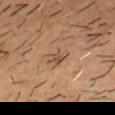The lesion was tiled from a total-body skin photograph and was not biopsied.
A male patient, roughly 50 years of age.
Longest diameter approximately 3 mm.
Cropped from a whole-body photographic skin survey; the tile spans about 15 mm.
The lesion is on the head or neck.
Automated tile analysis of the lesion measured an area of roughly 4.5 mm², an eccentricity of roughly 0.85, and two-axis asymmetry of about 0.25. It also reported a classifier nevus-likeness of about 0/100 and a detector confidence of about 100 out of 100 that the crop contains a lesion.